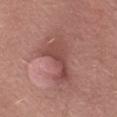The lesion was tiled from a total-body skin photograph and was not biopsied.
Imaged with white-light lighting.
Approximately 5.5 mm at its widest.
From the right thigh.
A 15 mm close-up tile from a total-body photography series done for melanoma screening.
Automated tile analysis of the lesion measured about 9 CIELAB-L* units darker than the surrounding skin. And it measured a border-irregularity index near 6.5/10 and a color-variation rating of about 3.5/10.
A male subject, aged around 60.